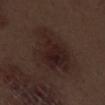Image and clinical context: A close-up tile cropped from a whole-body skin photograph, about 15 mm across. The patient is a male about 70 years old. Located on the left thigh. Automated image analysis of the tile measured a lesion color around L≈21 a*≈15 b*≈17 in CIELAB and about 6 CIELAB-L* units darker than the surrounding skin. About 7 mm across.